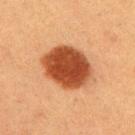Assessment:
No biopsy was performed on this lesion — it was imaged during a full skin examination and was not determined to be concerning.
Clinical summary:
A male subject, about 40 years old. Automated tile analysis of the lesion measured a lesion area of about 22 mm² and a symmetry-axis asymmetry near 0.1. The lesion is located on the right upper arm. A close-up tile cropped from a whole-body skin photograph, about 15 mm across. The recorded lesion diameter is about 5.5 mm. The tile uses cross-polarized illumination.